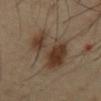The lesion was tiled from a total-body skin photograph and was not biopsied.